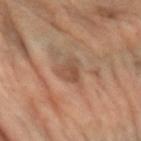lighting = cross-polarized | lesion diameter = about 3 mm | anatomic site = the arm | image-analysis metrics = a lesion color around L≈48 a*≈19 b*≈30 in CIELAB, roughly 8 lightness units darker than nearby skin, and a normalized lesion–skin contrast near 6; a border-irregularity rating of about 3.5/10, a within-lesion color-variation index near 4/10, and peripheral color asymmetry of about 1.5; a classifier nevus-likeness of about 0/100 | acquisition = ~15 mm tile from a whole-body skin photo | patient = female, aged 63–67.